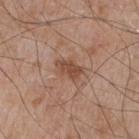workup: no biopsy performed (imaged during a skin exam) | tile lighting: white-light illumination | patient: male, aged 63 to 67 | lesion size: about 3.5 mm | location: the chest | image: 15 mm crop, total-body photography.